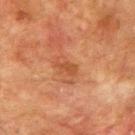biopsy status — catalogued during a skin exam; not biopsied
anatomic site — the right upper arm
lesion diameter — ~2.5 mm (longest diameter)
automated lesion analysis — a lesion–skin lightness drop of about 7
acquisition — 15 mm crop, total-body photography
subject — male, about 75 years old
lighting — cross-polarized